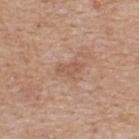{
  "site": "upper back",
  "patient": {
    "sex": "male",
    "age_approx": 60
  },
  "lesion_size": {
    "long_diameter_mm_approx": 3.0
  },
  "image": {
    "source": "total-body photography crop",
    "field_of_view_mm": 15
  },
  "automated_metrics": {
    "cielab_L": 55,
    "cielab_a": 20,
    "cielab_b": 29,
    "vs_skin_darker_L": 7.0,
    "vs_skin_contrast_norm": 5.5,
    "border_irregularity_0_10": 4.0,
    "color_variation_0_10": 1.0,
    "peripheral_color_asymmetry": 0.5,
    "nevus_likeness_0_100": 0,
    "lesion_detection_confidence_0_100": 100
  }
}